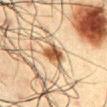notes = no biopsy performed (imaged during a skin exam)
subject = male, aged 58 to 62
acquisition = 15 mm crop, total-body photography
anatomic site = the chest
TBP lesion metrics = a lesion–skin lightness drop of about 15 and a normalized lesion–skin contrast near 12; a within-lesion color-variation index near 3.5/10 and peripheral color asymmetry of about 1.5; a classifier nevus-likeness of about 95/100 and a detector confidence of about 100 out of 100 that the crop contains a lesion